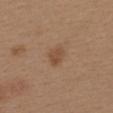Imaged during a routine full-body skin examination; the lesion was not biopsied and no histopathology is available. The subject is a female aged 38–42. A lesion tile, about 15 mm wide, cut from a 3D total-body photograph. The tile uses white-light illumination. The lesion is on the upper back. An algorithmic analysis of the crop reported internal color variation of about 1.5 on a 0–10 scale and a peripheral color-asymmetry measure near 0.5.